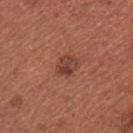Notes:
• follow-up · imaged on a skin check; not biopsied
• size · ≈2.5 mm
• tile lighting · white-light illumination
• subject · female, roughly 35 years of age
• automated metrics · an area of roughly 4.5 mm² and an eccentricity of roughly 0.65; a lesion color around L≈41 a*≈26 b*≈28 in CIELAB, a lesion–skin lightness drop of about 9, and a normalized border contrast of about 7; a border-irregularity rating of about 2/10, internal color variation of about 4 on a 0–10 scale, and a peripheral color-asymmetry measure near 1.5
• site · the arm
• imaging modality · 15 mm crop, total-body photography